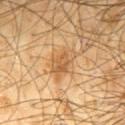Assessment:
Imaged during a routine full-body skin examination; the lesion was not biopsied and no histopathology is available.
Clinical summary:
A male patient, approximately 65 years of age. This is a cross-polarized tile. The lesion's longest dimension is about 4 mm. Located on the back. A roughly 15 mm field-of-view crop from a total-body skin photograph.